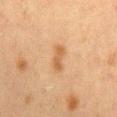workup — catalogued during a skin exam; not biopsied | acquisition — ~15 mm tile from a whole-body skin photo | automated metrics — a border-irregularity index near 3/10 and a color-variation rating of about 2/10 | site — the chest | illumination — cross-polarized illumination | subject — female, aged around 40 | diameter — about 3.5 mm.